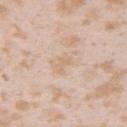Impression: The lesion was photographed on a routine skin check and not biopsied; there is no pathology result. Acquisition and patient details: A female subject, aged approximately 25. The lesion is located on the arm. A 15 mm close-up tile from a total-body photography series done for melanoma screening. This is a white-light tile.